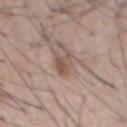workup = imaged on a skin check; not biopsied | tile lighting = white-light | subject = male, aged approximately 60 | image = ~15 mm crop, total-body skin-cancer survey | location = the chest.